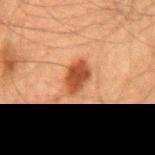<record>
  <biopsy_status>not biopsied; imaged during a skin examination</biopsy_status>
  <lighting>cross-polarized</lighting>
  <image>
    <source>total-body photography crop</source>
    <field_of_view_mm>15</field_of_view_mm>
  </image>
  <lesion_size>
    <long_diameter_mm_approx>4.0</long_diameter_mm_approx>
  </lesion_size>
  <site>back</site>
  <automated_metrics>
    <area_mm2_approx>7.5</area_mm2_approx>
    <eccentricity>0.8</eccentricity>
    <shape_asymmetry>0.2</shape_asymmetry>
    <nevus_likeness_0_100>90</nevus_likeness_0_100>
  </automated_metrics>
  <patient>
    <sex>male</sex>
    <age_approx>60</age_approx>
  </patient>
</record>